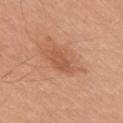Impression: Recorded during total-body skin imaging; not selected for excision or biopsy. Background: Automated tile analysis of the lesion measured an average lesion color of about L≈54 a*≈24 b*≈33 (CIELAB), about 8 CIELAB-L* units darker than the surrounding skin, and a normalized lesion–skin contrast near 5. The software also gave border irregularity of about 2 on a 0–10 scale and radial color variation of about 0.5. It also reported a classifier nevus-likeness of about 5/100 and a lesion-detection confidence of about 100/100. From the right upper arm. Approximately 3 mm at its widest. A roughly 15 mm field-of-view crop from a total-body skin photograph. Imaged with white-light lighting. The patient is a male in their 30s.